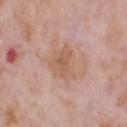notes = total-body-photography surveillance lesion; no biopsy | lesion diameter = ~4 mm (longest diameter) | anatomic site = the head or neck | automated lesion analysis = an automated nevus-likeness rating near 0 out of 100 | tile lighting = white-light | subject = male, aged 68–72 | imaging modality = ~15 mm tile from a whole-body skin photo.